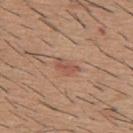{
  "biopsy_status": "not biopsied; imaged during a skin examination",
  "lesion_size": {
    "long_diameter_mm_approx": 3.0
  },
  "site": "upper back",
  "lighting": "white-light",
  "image": {
    "source": "total-body photography crop",
    "field_of_view_mm": 15
  },
  "patient": {
    "sex": "male",
    "age_approx": 60
  }
}